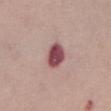No biopsy was performed on this lesion — it was imaged during a full skin examination and was not determined to be concerning. A female subject aged approximately 65. The tile uses white-light illumination. Automated tile analysis of the lesion measured a footprint of about 7 mm² and two-axis asymmetry of about 0.25. It also reported a normalized border contrast of about 12.5. And it measured border irregularity of about 2 on a 0–10 scale, a within-lesion color-variation index near 4/10, and radial color variation of about 1. Located on the chest. This image is a 15 mm lesion crop taken from a total-body photograph.